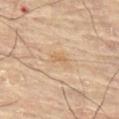Clinical impression:
Captured during whole-body skin photography for melanoma surveillance; the lesion was not biopsied.
Context:
The lesion is on the front of the torso. A female patient, in their 80s. A 15 mm close-up tile from a total-body photography series done for melanoma screening. The recorded lesion diameter is about 2.5 mm.